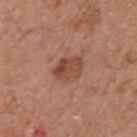Assessment:
This lesion was catalogued during total-body skin photography and was not selected for biopsy.
Context:
An algorithmic analysis of the crop reported an area of roughly 7 mm², a shape eccentricity near 0.7, and a symmetry-axis asymmetry near 0.25. And it measured a border-irregularity index near 2/10, a color-variation rating of about 5.5/10, and radial color variation of about 2. A male patient, aged around 65. The lesion is on the right upper arm. Captured under white-light illumination. A region of skin cropped from a whole-body photographic capture, roughly 15 mm wide. Approximately 3.5 mm at its widest.